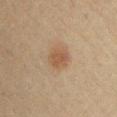Recorded during total-body skin imaging; not selected for excision or biopsy.
Approximately 2.5 mm at its widest.
The tile uses cross-polarized illumination.
The total-body-photography lesion software estimated roughly 7 lightness units darker than nearby skin and a normalized lesion–skin contrast near 6.5. And it measured border irregularity of about 1.5 on a 0–10 scale, internal color variation of about 2 on a 0–10 scale, and peripheral color asymmetry of about 0.5. And it measured lesion-presence confidence of about 100/100.
Cropped from a total-body skin-imaging series; the visible field is about 15 mm.
The lesion is on the chest.
A female subject, aged 38 to 42.